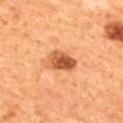Assessment: The lesion was photographed on a routine skin check and not biopsied; there is no pathology result. Image and clinical context: The lesion is on the upper back. A 15 mm crop from a total-body photograph taken for skin-cancer surveillance. An algorithmic analysis of the crop reported a lesion area of about 7 mm² and two-axis asymmetry of about 0.3. The software also gave a mean CIELAB color near L≈45 a*≈24 b*≈35 and a normalized border contrast of about 9.5. The software also gave a border-irregularity index near 3/10, a color-variation rating of about 6/10, and peripheral color asymmetry of about 2. The tile uses cross-polarized illumination. A female subject roughly 60 years of age. About 4 mm across.